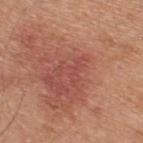Imaged during a routine full-body skin examination; the lesion was not biopsied and no histopathology is available.
A male patient approximately 45 years of age.
A close-up tile cropped from a whole-body skin photograph, about 15 mm across.
On the upper back.